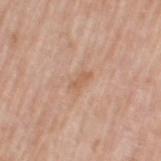The lesion was tiled from a total-body skin photograph and was not biopsied.
A female subject, aged 68 to 72.
A lesion tile, about 15 mm wide, cut from a 3D total-body photograph.
About 2.5 mm across.
From the left thigh.
Imaged with white-light lighting.